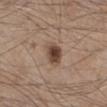Case summary:
– workup: no biopsy performed (imaged during a skin exam)
– illumination: white-light
– patient: male, about 45 years old
– image source: total-body-photography crop, ~15 mm field of view
– automated metrics: a lesion area of about 6.5 mm² and an outline eccentricity of about 0.65 (0 = round, 1 = elongated); a border-irregularity index near 1.5/10, internal color variation of about 5 on a 0–10 scale, and peripheral color asymmetry of about 1.5
– site: the right lower leg
– size: ≈3 mm